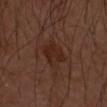<lesion>
<automated_metrics>
  <nevus_likeness_0_100>10</nevus_likeness_0_100>
</automated_metrics>
<site>arm</site>
<image>
  <source>total-body photography crop</source>
  <field_of_view_mm>15</field_of_view_mm>
</image>
<lighting>cross-polarized</lighting>
<lesion_size>
  <long_diameter_mm_approx>3.0</long_diameter_mm_approx>
</lesion_size>
</lesion>